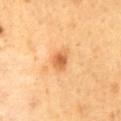Impression:
The lesion was tiled from a total-body skin photograph and was not biopsied.
Image and clinical context:
A male patient aged 48 to 52. The lesion-visualizer software estimated a mean CIELAB color near L≈65 a*≈27 b*≈45, a lesion–skin lightness drop of about 12, and a normalized lesion–skin contrast near 7.5. The software also gave a border-irregularity index near 3/10, internal color variation of about 3.5 on a 0–10 scale, and radial color variation of about 1. The software also gave an automated nevus-likeness rating near 60 out of 100. Captured under cross-polarized illumination. The lesion is located on the mid back. The lesion's longest dimension is about 3 mm. This image is a 15 mm lesion crop taken from a total-body photograph.